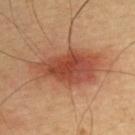No biopsy was performed on this lesion — it was imaged during a full skin examination and was not determined to be concerning.
A roughly 15 mm field-of-view crop from a total-body skin photograph.
The lesion is located on the upper back.
Longest diameter approximately 8.5 mm.
A male patient, aged around 65.
Automated image analysis of the tile measured an area of roughly 26 mm² and a shape-asymmetry score of about 0.25 (0 = symmetric). And it measured roughly 12 lightness units darker than nearby skin and a normalized border contrast of about 9. The analysis additionally found a border-irregularity rating of about 3.5/10 and radial color variation of about 1.5.
Imaged with cross-polarized lighting.